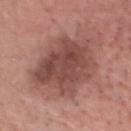notes: total-body-photography surveillance lesion; no biopsy
patient: male, approximately 75 years of age
location: the head or neck
lighting: white-light illumination
lesion size: ≈8 mm
image: ~15 mm crop, total-body skin-cancer survey